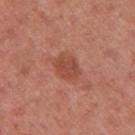Impression:
The lesion was tiled from a total-body skin photograph and was not biopsied.
Acquisition and patient details:
Measured at roughly 3.5 mm in maximum diameter. A region of skin cropped from a whole-body photographic capture, roughly 15 mm wide. On the right upper arm. A female patient roughly 50 years of age.